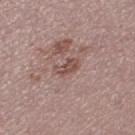Q: Was a biopsy performed?
A: no biopsy performed (imaged during a skin exam)
Q: What is the lesion's diameter?
A: ~3 mm (longest diameter)
Q: How was this image acquired?
A: 15 mm crop, total-body photography
Q: What did automated image analysis measure?
A: a lesion area of about 4 mm², an eccentricity of roughly 0.85, and a shape-asymmetry score of about 0.25 (0 = symmetric); border irregularity of about 2.5 on a 0–10 scale, internal color variation of about 2 on a 0–10 scale, and a peripheral color-asymmetry measure near 1; a nevus-likeness score of about 0/100
Q: Who is the patient?
A: female, aged 23–27
Q: How was the tile lit?
A: white-light illumination
Q: Where on the body is the lesion?
A: the left thigh